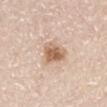Part of a total-body skin-imaging series; this lesion was reviewed on a skin check and was not flagged for biopsy.
On the left thigh.
A roughly 15 mm field-of-view crop from a total-body skin photograph.
The patient is a male about 80 years old.